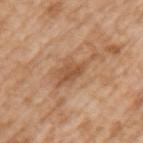Background: This is a white-light tile. The total-body-photography lesion software estimated a footprint of about 8.5 mm², an eccentricity of roughly 0.9, and a symmetry-axis asymmetry near 0.45. It also reported a lesion color around L≈54 a*≈21 b*≈35 in CIELAB, a lesion–skin lightness drop of about 10, and a normalized border contrast of about 6.5. And it measured a within-lesion color-variation index near 3.5/10 and peripheral color asymmetry of about 1.5. And it measured an automated nevus-likeness rating near 0 out of 100. A lesion tile, about 15 mm wide, cut from a 3D total-body photograph. The lesion is on the left upper arm. The subject is a male in their mid-60s. About 6 mm across.